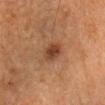workup — catalogued during a skin exam; not biopsied
image source — ~15 mm tile from a whole-body skin photo
tile lighting — cross-polarized illumination
location — the head or neck
patient — female, roughly 70 years of age
automated metrics — a footprint of about 5.5 mm² and a shape eccentricity near 0.4; a lesion-to-skin contrast of about 8 (normalized; higher = more distinct); internal color variation of about 2.5 on a 0–10 scale and peripheral color asymmetry of about 1; a nevus-likeness score of about 90/100 and a lesion-detection confidence of about 100/100
size — ~2.5 mm (longest diameter)